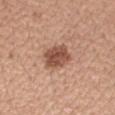Q: Is there a histopathology result?
A: catalogued during a skin exam; not biopsied
Q: What is the imaging modality?
A: total-body-photography crop, ~15 mm field of view
Q: Where on the body is the lesion?
A: the right upper arm
Q: Patient demographics?
A: female, in their mid-40s
Q: How large is the lesion?
A: about 4 mm
Q: Automated lesion metrics?
A: a shape eccentricity near 0.65 and a symmetry-axis asymmetry near 0.15; a lesion color around L≈51 a*≈22 b*≈29 in CIELAB, about 14 CIELAB-L* units darker than the surrounding skin, and a lesion-to-skin contrast of about 9.5 (normalized; higher = more distinct); internal color variation of about 4 on a 0–10 scale